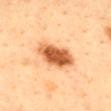Case summary:
- biopsy status: catalogued during a skin exam; not biopsied
- anatomic site: the back
- image: total-body-photography crop, ~15 mm field of view
- lighting: cross-polarized
- size: about 5 mm
- subject: male, roughly 40 years of age